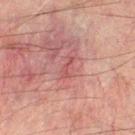follow-up = catalogued during a skin exam; not biopsied | site = the left leg | size = ≈2.5 mm | patient = male, aged 58 to 62 | illumination = cross-polarized | imaging modality = ~15 mm tile from a whole-body skin photo.